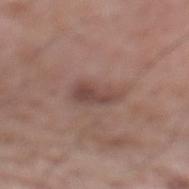notes = total-body-photography surveillance lesion; no biopsy
site = the mid back
subject = male, in their 60s
size = ≈4 mm
tile lighting = white-light
acquisition = ~15 mm tile from a whole-body skin photo